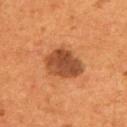Q: Is there a histopathology result?
A: no biopsy performed (imaged during a skin exam)
Q: How was the tile lit?
A: cross-polarized illumination
Q: What kind of image is this?
A: total-body-photography crop, ~15 mm field of view
Q: What is the anatomic site?
A: the back
Q: Automated lesion metrics?
A: a footprint of about 13 mm² and an outline eccentricity of about 0.75 (0 = round, 1 = elongated); a lesion color around L≈44 a*≈25 b*≈35 in CIELAB and a normalized border contrast of about 10; a classifier nevus-likeness of about 80/100 and a detector confidence of about 100 out of 100 that the crop contains a lesion
Q: Who is the patient?
A: male, approximately 55 years of age